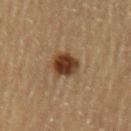Clinical impression:
The lesion was tiled from a total-body skin photograph and was not biopsied.
Acquisition and patient details:
On the left upper arm. The lesion-visualizer software estimated an eccentricity of roughly 0.4 and a shape-asymmetry score of about 0.2 (0 = symmetric). And it measured a mean CIELAB color near L≈33 a*≈17 b*≈28, a lesion–skin lightness drop of about 14, and a lesion-to-skin contrast of about 12.5 (normalized; higher = more distinct). The software also gave a border-irregularity rating of about 2/10 and a color-variation rating of about 4/10. About 3.5 mm across. A roughly 15 mm field-of-view crop from a total-body skin photograph. The tile uses cross-polarized illumination. A male patient aged around 60.